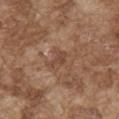<lesion>
  <site>front of the torso</site>
  <lighting>white-light</lighting>
  <image>
    <source>total-body photography crop</source>
    <field_of_view_mm>15</field_of_view_mm>
  </image>
  <patient>
    <sex>male</sex>
    <age_approx>75</age_approx>
  </patient>
</lesion>